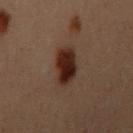workup=catalogued during a skin exam; not biopsied | location=the arm | size=about 3.5 mm | subject=female, aged 28–32 | image=~15 mm crop, total-body skin-cancer survey.